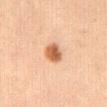notes: total-body-photography surveillance lesion; no biopsy | imaging modality: ~15 mm crop, total-body skin-cancer survey | patient: female, aged around 20 | anatomic site: the leg.